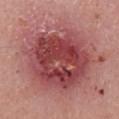Clinical summary:
A male patient about 70 years old. On the left lower leg. The tile uses white-light illumination. An algorithmic analysis of the crop reported a footprint of about 60 mm² and a symmetry-axis asymmetry near 0.2. It also reported a border-irregularity index near 3.5/10, a color-variation rating of about 8/10, and peripheral color asymmetry of about 2.5. The analysis additionally found a classifier nevus-likeness of about 0/100 and lesion-presence confidence of about 100/100. Cropped from a total-body skin-imaging series; the visible field is about 15 mm.
Conclusion:
On excision, pathology confirmed a malignancy: nodular basal cell carcinoma.